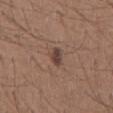  biopsy_status: not biopsied; imaged during a skin examination
  site: mid back
  lesion_size:
    long_diameter_mm_approx: 3.0
  lighting: white-light
  image:
    source: total-body photography crop
    field_of_view_mm: 15
  patient:
    sex: male
    age_approx: 25